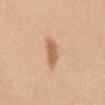  patient:
    sex: female
    age_approx: 30
  lesion_size:
    long_diameter_mm_approx: 3.5
  image:
    source: total-body photography crop
    field_of_view_mm: 15
  site: abdomen